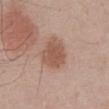Captured during whole-body skin photography for melanoma surveillance; the lesion was not biopsied. A male subject, about 50 years old. The tile uses white-light illumination. Cropped from a whole-body photographic skin survey; the tile spans about 15 mm. The lesion is located on the front of the torso.